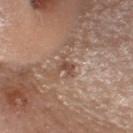Clinical summary: The lesion-visualizer software estimated a lesion–skin lightness drop of about 10 and a normalized border contrast of about 7. And it measured a border-irregularity rating of about 5.5/10, internal color variation of about 1.5 on a 0–10 scale, and a peripheral color-asymmetry measure near 0.5. The software also gave a classifier nevus-likeness of about 15/100 and a detector confidence of about 95 out of 100 that the crop contains a lesion. Located on the head or neck. Imaged with white-light lighting. Approximately 2.5 mm at its widest. A female subject, aged 63–67. A lesion tile, about 15 mm wide, cut from a 3D total-body photograph.